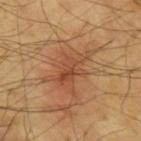The lesion was photographed on a routine skin check and not biopsied; there is no pathology result.
Captured under cross-polarized illumination.
The subject is a male aged around 65.
The lesion is located on the left forearm.
This image is a 15 mm lesion crop taken from a total-body photograph.